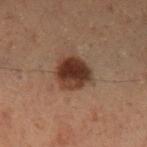The lesion was tiled from a total-body skin photograph and was not biopsied.